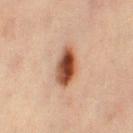biopsy status = total-body-photography surveillance lesion; no biopsy | patient = female, roughly 45 years of age | image = total-body-photography crop, ~15 mm field of view | diameter = ≈5 mm | lighting = cross-polarized | site = the leg.